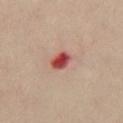Clinical impression: No biopsy was performed on this lesion — it was imaged during a full skin examination and was not determined to be concerning. Image and clinical context: Cropped from a total-body skin-imaging series; the visible field is about 15 mm. The subject is a female in their mid-50s. On the chest.